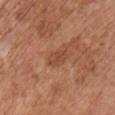Captured during whole-body skin photography for melanoma surveillance; the lesion was not biopsied.
Captured under white-light illumination.
The subject is a male in their mid-60s.
From the chest.
A 15 mm close-up tile from a total-body photography series done for melanoma screening.
About 3 mm across.
Automated image analysis of the tile measured a footprint of about 4 mm² and two-axis asymmetry of about 0.3. It also reported a lesion color around L≈47 a*≈24 b*≈33 in CIELAB, about 8 CIELAB-L* units darker than the surrounding skin, and a lesion-to-skin contrast of about 6 (normalized; higher = more distinct). It also reported a border-irregularity index near 2.5/10, a within-lesion color-variation index near 1.5/10, and peripheral color asymmetry of about 0.5. It also reported an automated nevus-likeness rating near 0 out of 100 and lesion-presence confidence of about 100/100.